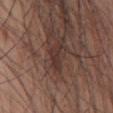| key | value |
|---|---|
| workup | total-body-photography surveillance lesion; no biopsy |
| lesion size | about 3.5 mm |
| image | total-body-photography crop, ~15 mm field of view |
| anatomic site | the front of the torso |
| lighting | white-light |
| patient | male, aged 53–57 |